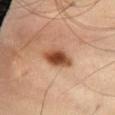<record>
<biopsy_status>not biopsied; imaged during a skin examination</biopsy_status>
<image>
  <source>total-body photography crop</source>
  <field_of_view_mm>15</field_of_view_mm>
</image>
<lesion_size>
  <long_diameter_mm_approx>4.0</long_diameter_mm_approx>
</lesion_size>
<site>abdomen</site>
<patient>
  <sex>male</sex>
  <age_approx>55</age_approx>
</patient>
<lighting>cross-polarized</lighting>
<automated_metrics>
  <area_mm2_approx>8.0</area_mm2_approx>
  <eccentricity>0.75</eccentricity>
  <shape_asymmetry>0.2</shape_asymmetry>
  <cielab_L>47</cielab_L>
  <cielab_a>23</cielab_a>
  <cielab_b>33</cielab_b>
  <vs_skin_darker_L>15.0</vs_skin_darker_L>
  <vs_skin_contrast_norm>11.0</vs_skin_contrast_norm>
  <border_irregularity_0_10>2.0</border_irregularity_0_10>
  <peripheral_color_asymmetry>1.5</peripheral_color_asymmetry>
  <lesion_detection_confidence_0_100>100</lesion_detection_confidence_0_100>
</automated_metrics>
</record>